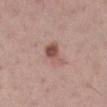Notes:
* biopsy status · imaged on a skin check; not biopsied
* illumination · white-light
* automated metrics · a footprint of about 6 mm² and a shape eccentricity near 0.75; a border-irregularity rating of about 4.5/10, a color-variation rating of about 5/10, and radial color variation of about 2; a classifier nevus-likeness of about 75/100 and a lesion-detection confidence of about 100/100
* image source · total-body-photography crop, ~15 mm field of view
* site · the right lower leg
* patient · female, aged approximately 25